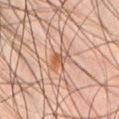Imaged during a routine full-body skin examination; the lesion was not biopsied and no histopathology is available. The lesion is located on the chest. The subject is a male in their mid- to late 40s. Imaged with cross-polarized lighting. A close-up tile cropped from a whole-body skin photograph, about 15 mm across.